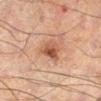This lesion was catalogued during total-body skin photography and was not selected for biopsy. Captured under cross-polarized illumination. A male subject aged around 60. A region of skin cropped from a whole-body photographic capture, roughly 15 mm wide. Longest diameter approximately 3.5 mm. The lesion is on the left leg.